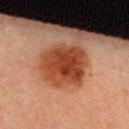The lesion was photographed on a routine skin check and not biopsied; there is no pathology result. A 15 mm close-up extracted from a 3D total-body photography capture. The recorded lesion diameter is about 5.5 mm. Captured under cross-polarized illumination. A female subject aged around 50. The total-body-photography lesion software estimated an outline eccentricity of about 0.45 (0 = round, 1 = elongated) and two-axis asymmetry of about 0.15. It also reported border irregularity of about 2 on a 0–10 scale, a within-lesion color-variation index near 6.5/10, and peripheral color asymmetry of about 2. It also reported an automated nevus-likeness rating near 100 out of 100 and lesion-presence confidence of about 100/100.